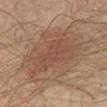Q: Is there a histopathology result?
A: imaged on a skin check; not biopsied
Q: What are the patient's age and sex?
A: in their mid-50s
Q: Lesion location?
A: the chest
Q: How was this image acquired?
A: ~15 mm crop, total-body skin-cancer survey